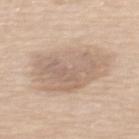No biopsy was performed on this lesion — it was imaged during a full skin examination and was not determined to be concerning.
This image is a 15 mm lesion crop taken from a total-body photograph.
A female subject, roughly 65 years of age.
Captured under white-light illumination.
Longest diameter approximately 7.5 mm.
The total-body-photography lesion software estimated a lesion-detection confidence of about 100/100.
From the upper back.